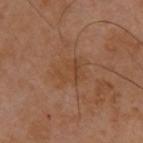Impression:
The lesion was tiled from a total-body skin photograph and was not biopsied.
Background:
The lesion's longest dimension is about 4 mm. The lesion is on the upper back. An algorithmic analysis of the crop reported border irregularity of about 3.5 on a 0–10 scale and a peripheral color-asymmetry measure near 1. This is a cross-polarized tile. The patient is a male aged around 40. A roughly 15 mm field-of-view crop from a total-body skin photograph.